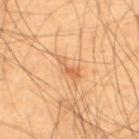Part of a total-body skin-imaging series; this lesion was reviewed on a skin check and was not flagged for biopsy. This image is a 15 mm lesion crop taken from a total-body photograph. The lesion is on the upper back. A male subject aged around 55. An algorithmic analysis of the crop reported a footprint of about 4 mm², an outline eccentricity of about 0.9 (0 = round, 1 = elongated), and two-axis asymmetry of about 0.45. And it measured a lesion color around L≈63 a*≈24 b*≈42 in CIELAB and roughly 9 lightness units darker than nearby skin. It also reported a classifier nevus-likeness of about 5/100 and lesion-presence confidence of about 100/100. Approximately 3.5 mm at its widest. Imaged with cross-polarized lighting.